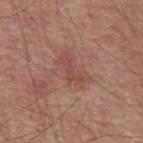notes = catalogued during a skin exam; not biopsied
tile lighting = white-light
lesion size = ≈3 mm
subject = male, about 55 years old
image = 15 mm crop, total-body photography
image-analysis metrics = a lesion color around L≈46 a*≈24 b*≈25 in CIELAB, a lesion–skin lightness drop of about 7, and a normalized border contrast of about 5; a border-irregularity rating of about 8/10 and a within-lesion color-variation index near 0/10
location = the mid back